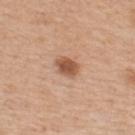biopsy_status: not biopsied; imaged during a skin examination
patient:
  sex: male
  age_approx: 65
site: upper back
automated_metrics:
  area_mm2_approx: 5.5
  eccentricity: 0.7
  shape_asymmetry: 0.15
  peripheral_color_asymmetry: 1.0
  nevus_likeness_0_100: 95
image:
  source: total-body photography crop
  field_of_view_mm: 15
lighting: white-light